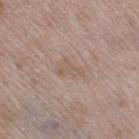biopsy status: total-body-photography surveillance lesion; no biopsy
patient: female, in their 50s
lighting: white-light
size: about 3 mm
anatomic site: the leg
acquisition: total-body-photography crop, ~15 mm field of view
automated lesion analysis: a lesion area of about 4 mm², an eccentricity of roughly 0.8, and a shape-asymmetry score of about 0.5 (0 = symmetric); a border-irregularity index near 5/10, a within-lesion color-variation index near 1/10, and a peripheral color-asymmetry measure near 0.5; a nevus-likeness score of about 0/100 and a detector confidence of about 100 out of 100 that the crop contains a lesion